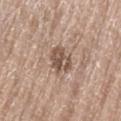Clinical impression: Captured during whole-body skin photography for melanoma surveillance; the lesion was not biopsied. Acquisition and patient details: A 15 mm close-up extracted from a 3D total-body photography capture. From the lower back. A male subject aged around 80.